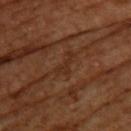The lesion was tiled from a total-body skin photograph and was not biopsied. Approximately 3 mm at its widest. The lesion is located on the upper back. A 15 mm close-up extracted from a 3D total-body photography capture. The subject is a female aged 53 to 57.